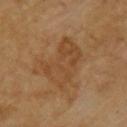The lesion was tiled from a total-body skin photograph and was not biopsied. The recorded lesion diameter is about 5.5 mm. The patient is a male aged 63–67. Automated image analysis of the tile measured a lesion color around L≈45 a*≈19 b*≈35 in CIELAB and about 6 CIELAB-L* units darker than the surrounding skin. The lesion is on the chest. A lesion tile, about 15 mm wide, cut from a 3D total-body photograph.